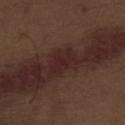biopsy_status: not biopsied; imaged during a skin examination
lighting: white-light
site: abdomen
image:
  source: total-body photography crop
  field_of_view_mm: 15
patient:
  sex: male
  age_approx: 70